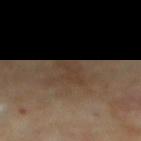Recorded during total-body skin imaging; not selected for excision or biopsy.
Located on the mid back.
The subject is a male aged around 85 or older.
Measured at roughly 3 mm in maximum diameter.
A 15 mm crop from a total-body photograph taken for skin-cancer surveillance.
Captured under cross-polarized illumination.